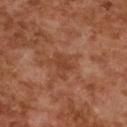| field | value |
|---|---|
| biopsy status | catalogued during a skin exam; not biopsied |
| acquisition | total-body-photography crop, ~15 mm field of view |
| lesion diameter | ≈3 mm |
| lighting | cross-polarized illumination |
| patient | female, about 60 years old |
| body site | the upper back |